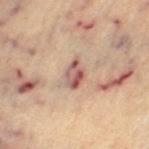The lesion was photographed on a routine skin check and not biopsied; there is no pathology result. A 15 mm crop from a total-body photograph taken for skin-cancer surveillance. The lesion is on the left thigh. The patient is a female approximately 65 years of age.